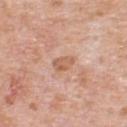notes = imaged on a skin check; not biopsied | tile lighting = white-light | image = 15 mm crop, total-body photography | location = the back | patient = male, aged approximately 80 | TBP lesion metrics = a nevus-likeness score of about 0/100 and lesion-presence confidence of about 100/100 | lesion size = about 3 mm.